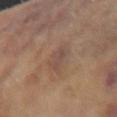A male subject, aged 58 to 62.
A close-up tile cropped from a whole-body skin photograph, about 15 mm across.
Captured under white-light illumination.
On the right forearm.
The lesion-visualizer software estimated a footprint of about 6 mm² and a symmetry-axis asymmetry near 0.35. The analysis additionally found an average lesion color of about L≈48 a*≈17 b*≈25 (CIELAB), roughly 6 lightness units darker than nearby skin, and a lesion-to-skin contrast of about 5.5 (normalized; higher = more distinct). The analysis additionally found an automated nevus-likeness rating near 0 out of 100.